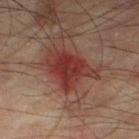Captured during whole-body skin photography for melanoma surveillance; the lesion was not biopsied. Imaged with cross-polarized lighting. On the leg. Automated tile analysis of the lesion measured border irregularity of about 4.5 on a 0–10 scale. Longest diameter approximately 5 mm. A male subject, approximately 75 years of age. A roughly 15 mm field-of-view crop from a total-body skin photograph.